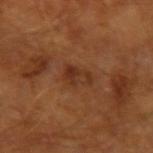{"patient": {"sex": "male", "age_approx": 65}, "site": "left forearm", "image": {"source": "total-body photography crop", "field_of_view_mm": 15}}